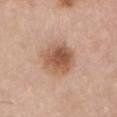Part of a total-body skin-imaging series; this lesion was reviewed on a skin check and was not flagged for biopsy. Cropped from a whole-body photographic skin survey; the tile spans about 15 mm. Located on the chest. The subject is a male aged approximately 75. This is a white-light tile. The recorded lesion diameter is about 5 mm.